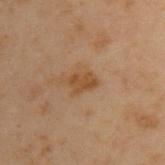| key | value |
|---|---|
| notes | catalogued during a skin exam; not biopsied |